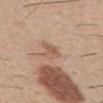Imaged during a routine full-body skin examination; the lesion was not biopsied and no histopathology is available.
Located on the abdomen.
The recorded lesion diameter is about 2.5 mm.
The subject is a male in their 30s.
A 15 mm close-up extracted from a 3D total-body photography capture.